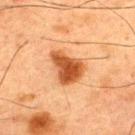The lesion was tiled from a total-body skin photograph and was not biopsied.